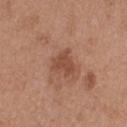| field | value |
|---|---|
| workup | no biopsy performed (imaged during a skin exam) |
| location | the left thigh |
| illumination | white-light illumination |
| diameter | about 3 mm |
| acquisition | total-body-photography crop, ~15 mm field of view |
| patient | female, aged 38–42 |